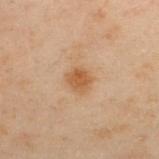Q: Is there a histopathology result?
A: no biopsy performed (imaged during a skin exam)
Q: What did automated image analysis measure?
A: a lesion color around L≈46 a*≈18 b*≈32 in CIELAB and about 8 CIELAB-L* units darker than the surrounding skin; a border-irregularity rating of about 2/10 and radial color variation of about 0.5; a lesion-detection confidence of about 100/100
Q: Lesion size?
A: about 2.5 mm
Q: Patient demographics?
A: male, about 50 years old
Q: How was this image acquired?
A: 15 mm crop, total-body photography
Q: What is the anatomic site?
A: the upper back